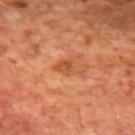On the mid back. This image is a 15 mm lesion crop taken from a total-body photograph. A male subject aged approximately 70. Measured at roughly 3.5 mm in maximum diameter. Captured under cross-polarized illumination.